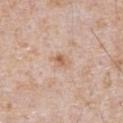The lesion was photographed on a routine skin check and not biopsied; there is no pathology result. The total-body-photography lesion software estimated an outline eccentricity of about 0.7 (0 = round, 1 = elongated) and a shape-asymmetry score of about 0.2 (0 = symmetric). It also reported a nevus-likeness score of about 5/100. Captured under white-light illumination. The subject is a male about 65 years old. About 2.5 mm across. A lesion tile, about 15 mm wide, cut from a 3D total-body photograph. Located on the abdomen.